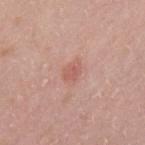Impression:
Imaged during a routine full-body skin examination; the lesion was not biopsied and no histopathology is available.
Context:
An algorithmic analysis of the crop reported a footprint of about 4 mm², an eccentricity of roughly 0.75, and a shape-asymmetry score of about 0.25 (0 = symmetric). And it measured a lesion color around L≈58 a*≈25 b*≈26 in CIELAB, roughly 8 lightness units darker than nearby skin, and a normalized lesion–skin contrast near 5.5. And it measured a classifier nevus-likeness of about 5/100 and a detector confidence of about 100 out of 100 that the crop contains a lesion. A 15 mm crop from a total-body photograph taken for skin-cancer surveillance. Longest diameter approximately 2.5 mm. The patient is a female aged 28 to 32. This is a white-light tile. The lesion is located on the upper back.